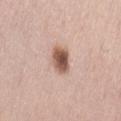Impression: Captured during whole-body skin photography for melanoma surveillance; the lesion was not biopsied. Acquisition and patient details: From the left thigh. The recorded lesion diameter is about 3.5 mm. This is a white-light tile. A 15 mm close-up extracted from a 3D total-body photography capture. A female patient aged around 50. Automated image analysis of the tile measured an average lesion color of about L≈55 a*≈20 b*≈27 (CIELAB), a lesion–skin lightness drop of about 17, and a normalized lesion–skin contrast near 11. And it measured a border-irregularity rating of about 1.5/10 and internal color variation of about 5.5 on a 0–10 scale. The software also gave an automated nevus-likeness rating near 100 out of 100.